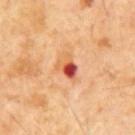The lesion was tiled from a total-body skin photograph and was not biopsied. A male subject, roughly 65 years of age. The lesion's longest dimension is about 3 mm. This image is a 15 mm lesion crop taken from a total-body photograph. The tile uses cross-polarized illumination.